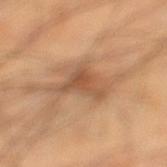Part of a total-body skin-imaging series; this lesion was reviewed on a skin check and was not flagged for biopsy. A 15 mm crop from a total-body photograph taken for skin-cancer surveillance. The lesion is located on the left lower leg. Approximately 5.5 mm at its widest. A male patient, aged approximately 40. Captured under cross-polarized illumination.